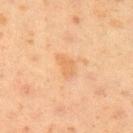Findings:
* follow-up: no biopsy performed (imaged during a skin exam)
* imaging modality: 15 mm crop, total-body photography
* patient: female, aged 18 to 22
* location: the arm